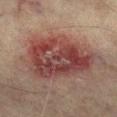Impression: Captured during whole-body skin photography for melanoma surveillance; the lesion was not biopsied. Acquisition and patient details: The patient is a male in their mid-70s. Imaged with cross-polarized lighting. Automated tile analysis of the lesion measured a footprint of about 38 mm², a shape eccentricity near 0.75, and two-axis asymmetry of about 0.25. And it measured a lesion color around L≈35 a*≈21 b*≈20 in CIELAB and a normalized border contrast of about 10. The lesion is located on the right lower leg. A close-up tile cropped from a whole-body skin photograph, about 15 mm across. Longest diameter approximately 9 mm.